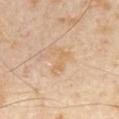Findings:
• biopsy status — total-body-photography surveillance lesion; no biopsy
• acquisition — ~15 mm crop, total-body skin-cancer survey
• subject — male, aged around 65
• lesion size — ~3.5 mm (longest diameter)
• lighting — cross-polarized